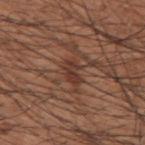Case summary:
– biopsy status — imaged on a skin check; not biopsied
– body site — the upper back
– patient — male, approximately 60 years of age
– automated lesion analysis — an area of roughly 4.5 mm² and a symmetry-axis asymmetry near 0.35; roughly 8 lightness units darker than nearby skin; border irregularity of about 4.5 on a 0–10 scale, a within-lesion color-variation index near 3/10, and peripheral color asymmetry of about 1; an automated nevus-likeness rating near 0 out of 100 and lesion-presence confidence of about 95/100
– image source — ~15 mm crop, total-body skin-cancer survey
– lighting — white-light illumination